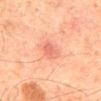Clinical impression:
The lesion was photographed on a routine skin check and not biopsied; there is no pathology result.
Background:
A 15 mm crop from a total-body photograph taken for skin-cancer surveillance. The lesion is on the mid back. Automated tile analysis of the lesion measured an average lesion color of about L≈53 a*≈26 b*≈28 (CIELAB) and about 8 CIELAB-L* units darker than the surrounding skin. The analysis additionally found a within-lesion color-variation index near 3/10 and peripheral color asymmetry of about 1. And it measured a nevus-likeness score of about 20/100. Captured under cross-polarized illumination. The patient is a male aged approximately 65.